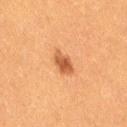The lesion was tiled from a total-body skin photograph and was not biopsied. An algorithmic analysis of the crop reported internal color variation of about 3 on a 0–10 scale and radial color variation of about 1. The subject is a female in their 40s. The tile uses cross-polarized illumination. The lesion is on the right thigh. About 3 mm across. A close-up tile cropped from a whole-body skin photograph, about 15 mm across.